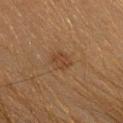Assessment: The lesion was photographed on a routine skin check and not biopsied; there is no pathology result. Background: The tile uses cross-polarized illumination. The total-body-photography lesion software estimated a lesion–skin lightness drop of about 6 and a normalized border contrast of about 5.5. And it measured border irregularity of about 3 on a 0–10 scale, internal color variation of about 2.5 on a 0–10 scale, and a peripheral color-asymmetry measure near 1. And it measured a nevus-likeness score of about 5/100 and a lesion-detection confidence of about 100/100. A 15 mm close-up tile from a total-body photography series done for melanoma screening. The subject is a female approximately 20 years of age. From the head or neck.